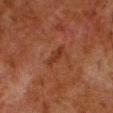Clinical impression: Part of a total-body skin-imaging series; this lesion was reviewed on a skin check and was not flagged for biopsy. Image and clinical context: The lesion is located on the left lower leg. A male patient, about 80 years old. A close-up tile cropped from a whole-body skin photograph, about 15 mm across.